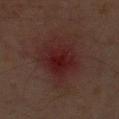Captured under cross-polarized illumination. A 15 mm close-up extracted from a 3D total-body photography capture. The recorded lesion diameter is about 5.5 mm. The subject is a male roughly 60 years of age. Located on the left upper arm. Automated tile analysis of the lesion measured a footprint of about 24 mm², an eccentricity of roughly 0.3, and two-axis asymmetry of about 0.2. The analysis additionally found an automated nevus-likeness rating near 0 out of 100 and a detector confidence of about 100 out of 100 that the crop contains a lesion.